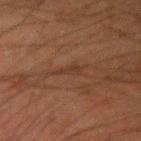| key | value |
|---|---|
| notes | no biopsy performed (imaged during a skin exam) |
| patient | male, aged 33 to 37 |
| image | total-body-photography crop, ~15 mm field of view |
| location | the left forearm |
| lesion diameter | ≈2.5 mm |